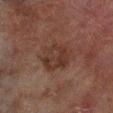follow-up: imaged on a skin check; not biopsied
lesion diameter: about 5 mm
image: ~15 mm tile from a whole-body skin photo
site: the leg
subject: male, roughly 70 years of age